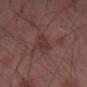Imaged during a routine full-body skin examination; the lesion was not biopsied and no histopathology is available. Approximately 3.5 mm at its widest. Automated tile analysis of the lesion measured a footprint of about 3.5 mm² and an eccentricity of roughly 0.95. Cropped from a total-body skin-imaging series; the visible field is about 15 mm. Imaged with white-light lighting. The lesion is on the left thigh. A male subject in their 50s.